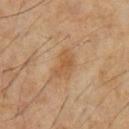  automated_metrics:
    vs_skin_darker_L: 7.0
    nevus_likeness_0_100: 0
    lesion_detection_confidence_0_100: 100
  lesion_size:
    long_diameter_mm_approx: 3.5
  site: chest
  lighting: cross-polarized
  image:
    source: total-body photography crop
    field_of_view_mm: 15
  patient:
    sex: male
    age_approx: 60